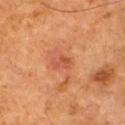Clinical impression: Part of a total-body skin-imaging series; this lesion was reviewed on a skin check and was not flagged for biopsy. Clinical summary: Longest diameter approximately 2.5 mm. The patient is a male aged approximately 60. From the left arm. This image is a 15 mm lesion crop taken from a total-body photograph.